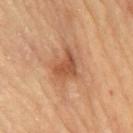<tbp_lesion>
  <patient>
    <sex>male</sex>
    <age_approx>85+</age_approx>
  </patient>
  <image>
    <source>total-body photography crop</source>
    <field_of_view_mm>15</field_of_view_mm>
  </image>
  <site>chest</site>
</tbp_lesion>